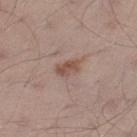Q: Was a biopsy performed?
A: catalogued during a skin exam; not biopsied
Q: What is the anatomic site?
A: the left thigh
Q: What kind of image is this?
A: 15 mm crop, total-body photography
Q: Who is the patient?
A: male, in their mid- to late 30s